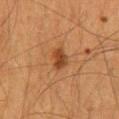Q: Is there a histopathology result?
A: no biopsy performed (imaged during a skin exam)
Q: What is the anatomic site?
A: the mid back
Q: What are the patient's age and sex?
A: male, aged approximately 65
Q: Illumination type?
A: cross-polarized illumination
Q: What is the imaging modality?
A: ~15 mm tile from a whole-body skin photo
Q: What is the lesion's diameter?
A: ~2.5 mm (longest diameter)